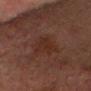Imaged during a routine full-body skin examination; the lesion was not biopsied and no histopathology is available.
This image is a 15 mm lesion crop taken from a total-body photograph.
The subject is a male in their mid-70s.
The lesion is located on the head or neck.